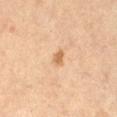A roughly 15 mm field-of-view crop from a total-body skin photograph.
The subject is a female approximately 65 years of age.
Automated image analysis of the tile measured a within-lesion color-variation index near 0.5/10 and peripheral color asymmetry of about 0. And it measured an automated nevus-likeness rating near 70 out of 100 and a detector confidence of about 100 out of 100 that the crop contains a lesion.
From the leg.
Longest diameter approximately 2 mm.